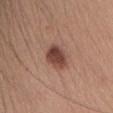  biopsy_status: not biopsied; imaged during a skin examination
  patient:
    sex: female
    age_approx: 35
  lighting: white-light
  site: head or neck
  lesion_size:
    long_diameter_mm_approx: 3.5
  image:
    source: total-body photography crop
    field_of_view_mm: 15
  automated_metrics:
    nevus_likeness_0_100: 90
    lesion_detection_confidence_0_100: 100